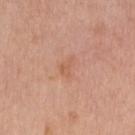Assessment: No biopsy was performed on this lesion — it was imaged during a full skin examination and was not determined to be concerning. Background: The patient is a female in their mid-60s. The recorded lesion diameter is about 3 mm. Automated tile analysis of the lesion measured a lesion color around L≈60 a*≈23 b*≈33 in CIELAB and a normalized border contrast of about 4.5. The software also gave a border-irregularity rating of about 4.5/10, a within-lesion color-variation index near 1/10, and peripheral color asymmetry of about 0.5. A roughly 15 mm field-of-view crop from a total-body skin photograph. On the right upper arm. Captured under white-light illumination.